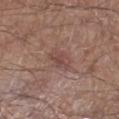No biopsy was performed on this lesion — it was imaged during a full skin examination and was not determined to be concerning.
A male patient aged 53–57.
From the right lower leg.
The tile uses white-light illumination.
The lesion-visualizer software estimated a border-irregularity index near 2.5/10 and a within-lesion color-variation index near 3/10.
Measured at roughly 3.5 mm in maximum diameter.
A roughly 15 mm field-of-view crop from a total-body skin photograph.